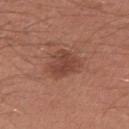Notes:
* anatomic site — the arm
* lighting — white-light illumination
* subject — male, roughly 30 years of age
* image — ~15 mm crop, total-body skin-cancer survey
* size — about 4 mm